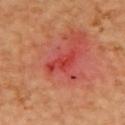No biopsy was performed on this lesion — it was imaged during a full skin examination and was not determined to be concerning.